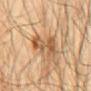workup = total-body-photography surveillance lesion; no biopsy
body site = the mid back
image source = ~15 mm tile from a whole-body skin photo
subject = male, aged approximately 65
lighting = cross-polarized
lesion size = ~6.5 mm (longest diameter)